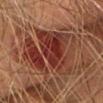The lesion was photographed on a routine skin check and not biopsied; there is no pathology result. A female subject roughly 60 years of age. The lesion is located on the head or neck. A roughly 15 mm field-of-view crop from a total-body skin photograph. The recorded lesion diameter is about 7.5 mm.